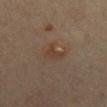Captured during whole-body skin photography for melanoma surveillance; the lesion was not biopsied. The patient is a female aged approximately 65. Cropped from a whole-body photographic skin survey; the tile spans about 15 mm. From the abdomen. The tile uses cross-polarized illumination.